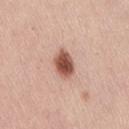* workup · catalogued during a skin exam; not biopsied
* location · the lower back
* image-analysis metrics · a mean CIELAB color near L≈53 a*≈24 b*≈28 and a normalized border contrast of about 11.5; an automated nevus-likeness rating near 100 out of 100 and lesion-presence confidence of about 100/100
* patient · female, about 50 years old
* image source · 15 mm crop, total-body photography
* illumination · white-light